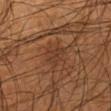<case>
  <biopsy_status>not biopsied; imaged during a skin examination</biopsy_status>
  <automated_metrics>
    <area_mm2_approx>4.0</area_mm2_approx>
    <shape_asymmetry>0.4</shape_asymmetry>
    <color_variation_0_10>0.5</color_variation_0_10>
    <peripheral_color_asymmetry>0.0</peripheral_color_asymmetry>
    <nevus_likeness_0_100>0</nevus_likeness_0_100>
    <lesion_detection_confidence_0_100>65</lesion_detection_confidence_0_100>
  </automated_metrics>
  <image>
    <source>total-body photography crop</source>
    <field_of_view_mm>15</field_of_view_mm>
  </image>
  <lesion_size>
    <long_diameter_mm_approx>3.0</long_diameter_mm_approx>
  </lesion_size>
  <lighting>cross-polarized</lighting>
  <patient>
    <sex>male</sex>
    <age_approx>55</age_approx>
  </patient>
  <site>leg</site>
</case>